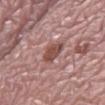Imaged during a routine full-body skin examination; the lesion was not biopsied and no histopathology is available. On the leg. A roughly 15 mm field-of-view crop from a total-body skin photograph. The patient is a female aged approximately 65. Imaged with white-light lighting. The lesion-visualizer software estimated a border-irregularity rating of about 2.5/10, internal color variation of about 3 on a 0–10 scale, and a peripheral color-asymmetry measure near 1. The recorded lesion diameter is about 3.5 mm.